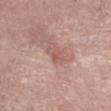The lesion was tiled from a total-body skin photograph and was not biopsied.
On the left lower leg.
Cropped from a total-body skin-imaging series; the visible field is about 15 mm.
The tile uses white-light illumination.
The lesion's longest dimension is about 2.5 mm.
A female patient approximately 65 years of age.
The lesion-visualizer software estimated an average lesion color of about L≈56 a*≈23 b*≈24 (CIELAB), roughly 8 lightness units darker than nearby skin, and a normalized border contrast of about 5.5. And it measured a border-irregularity index near 4.5/10, a color-variation rating of about 0/10, and a peripheral color-asymmetry measure near 0. The analysis additionally found an automated nevus-likeness rating near 5 out of 100 and a detector confidence of about 85 out of 100 that the crop contains a lesion.